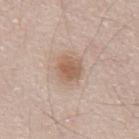The lesion was photographed on a routine skin check and not biopsied; there is no pathology result.
A lesion tile, about 15 mm wide, cut from a 3D total-body photograph.
Longest diameter approximately 3 mm.
Imaged with white-light lighting.
The subject is a male aged approximately 65.
The lesion-visualizer software estimated a mean CIELAB color near L≈58 a*≈18 b*≈29, roughly 10 lightness units darker than nearby skin, and a normalized border contrast of about 7.5.
The lesion is located on the abdomen.